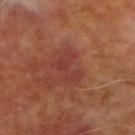Impression: Imaged during a routine full-body skin examination; the lesion was not biopsied and no histopathology is available. Image and clinical context: A male patient, aged 68 to 72. This is a cross-polarized tile. Automated tile analysis of the lesion measured an area of roughly 6 mm², an outline eccentricity of about 0.75 (0 = round, 1 = elongated), and a shape-asymmetry score of about 0.55 (0 = symmetric). It also reported an average lesion color of about L≈31 a*≈23 b*≈23 (CIELAB), roughly 5 lightness units darker than nearby skin, and a normalized lesion–skin contrast near 5. The software also gave a border-irregularity rating of about 8/10, a within-lesion color-variation index near 1.5/10, and peripheral color asymmetry of about 0.5. The analysis additionally found a classifier nevus-likeness of about 0/100 and lesion-presence confidence of about 100/100. The lesion is located on the right forearm. A lesion tile, about 15 mm wide, cut from a 3D total-body photograph. The lesion's longest dimension is about 4 mm.